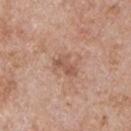– size · ~3 mm (longest diameter)
– body site · the chest
– patient · male, roughly 65 years of age
– image-analysis metrics · an eccentricity of roughly 0.85; a lesion color around L≈55 a*≈21 b*≈29 in CIELAB and a lesion-to-skin contrast of about 6 (normalized; higher = more distinct); a border-irregularity index near 4/10, a within-lesion color-variation index near 3/10, and peripheral color asymmetry of about 1
– imaging modality · ~15 mm crop, total-body skin-cancer survey
– illumination · white-light